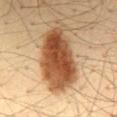Q: Was a biopsy performed?
A: no biopsy performed (imaged during a skin exam)
Q: How was the tile lit?
A: cross-polarized
Q: What is the anatomic site?
A: the back
Q: Patient demographics?
A: male, aged 33–37
Q: What kind of image is this?
A: 15 mm crop, total-body photography